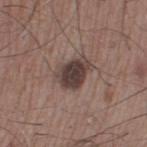Impression:
Recorded during total-body skin imaging; not selected for excision or biopsy.
Context:
The patient is a male roughly 50 years of age. A lesion tile, about 15 mm wide, cut from a 3D total-body photograph. Located on the left thigh.